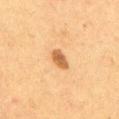{
  "biopsy_status": "not biopsied; imaged during a skin examination",
  "patient": {
    "sex": "female",
    "age_approx": 50
  },
  "image": {
    "source": "total-body photography crop",
    "field_of_view_mm": 15
  },
  "lighting": "cross-polarized",
  "automated_metrics": {
    "area_mm2_approx": 3.5,
    "eccentricity": 0.8,
    "shape_asymmetry": 0.25,
    "cielab_L": 50,
    "cielab_a": 20,
    "cielab_b": 36,
    "vs_skin_darker_L": 13.0,
    "vs_skin_contrast_norm": 9.0,
    "nevus_likeness_0_100": 95,
    "lesion_detection_confidence_0_100": 100
  },
  "site": "abdomen",
  "lesion_size": {
    "long_diameter_mm_approx": 3.0
  }
}